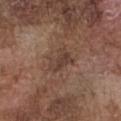Imaged during a routine full-body skin examination; the lesion was not biopsied and no histopathology is available. About 3.5 mm across. An algorithmic analysis of the crop reported a lesion color around L≈40 a*≈18 b*≈24 in CIELAB, roughly 7 lightness units darker than nearby skin, and a lesion-to-skin contrast of about 6.5 (normalized; higher = more distinct). And it measured a border-irregularity index near 3.5/10, a within-lesion color-variation index near 3/10, and peripheral color asymmetry of about 1. The software also gave a classifier nevus-likeness of about 0/100 and a lesion-detection confidence of about 100/100. Located on the chest. A region of skin cropped from a whole-body photographic capture, roughly 15 mm wide. A male subject about 75 years old. This is a white-light tile.